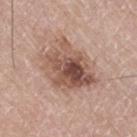<record>
  <biopsy_status>not biopsied; imaged during a skin examination</biopsy_status>
  <lighting>white-light</lighting>
  <patient>
    <sex>male</sex>
    <age_approx>75</age_approx>
  </patient>
  <site>right thigh</site>
  <lesion_size>
    <long_diameter_mm_approx>6.5</long_diameter_mm_approx>
  </lesion_size>
  <image>
    <source>total-body photography crop</source>
    <field_of_view_mm>15</field_of_view_mm>
  </image>
</record>